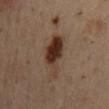Part of a total-body skin-imaging series; this lesion was reviewed on a skin check and was not flagged for biopsy. A male patient aged approximately 55. A lesion tile, about 15 mm wide, cut from a 3D total-body photograph. From the mid back. An algorithmic analysis of the crop reported a footprint of about 12 mm², an eccentricity of roughly 0.95, and a shape-asymmetry score of about 0.3 (0 = symmetric). It also reported an average lesion color of about L≈32 a*≈16 b*≈24 (CIELAB) and a lesion–skin lightness drop of about 12. Measured at roughly 7 mm in maximum diameter. Captured under cross-polarized illumination.